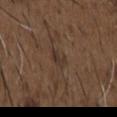Clinical impression: The lesion was photographed on a routine skin check and not biopsied; there is no pathology result. Image and clinical context: Imaged with white-light lighting. A male patient about 50 years old. A 15 mm close-up tile from a total-body photography series done for melanoma screening. Longest diameter approximately 2.5 mm. Located on the chest. The total-body-photography lesion software estimated an area of roughly 3 mm² and a symmetry-axis asymmetry near 0.4. The analysis additionally found a border-irregularity rating of about 3.5/10 and a within-lesion color-variation index near 0.5/10. And it measured a detector confidence of about 75 out of 100 that the crop contains a lesion.